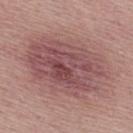This lesion was catalogued during total-body skin photography and was not selected for biopsy. About 10 mm across. The patient is a male roughly 30 years of age. A 15 mm crop from a total-body photograph taken for skin-cancer surveillance.